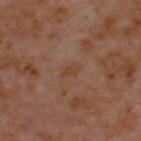Recorded during total-body skin imaging; not selected for excision or biopsy. A 15 mm close-up extracted from a 3D total-body photography capture. The lesion is on the upper back. A male subject about 60 years old.